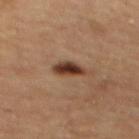subject: female, approximately 60 years of age
image-analysis metrics: a mean CIELAB color near L≈35 a*≈19 b*≈27, roughly 14 lightness units darker than nearby skin, and a normalized border contrast of about 12; border irregularity of about 2.5 on a 0–10 scale, a color-variation rating of about 6/10, and a peripheral color-asymmetry measure near 2; lesion-presence confidence of about 100/100
site: the mid back
image: total-body-photography crop, ~15 mm field of view